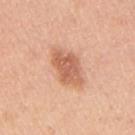biopsy_status: not biopsied; imaged during a skin examination
lesion_size:
  long_diameter_mm_approx: 5.0
lighting: white-light
patient:
  sex: male
  age_approx: 50
image:
  source: total-body photography crop
  field_of_view_mm: 15
automated_metrics:
  area_mm2_approx: 11.0
  eccentricity: 0.8
  shape_asymmetry: 0.25
  cielab_L: 62
  cielab_a: 25
  cielab_b: 34
  vs_skin_darker_L: 12.0
site: arm